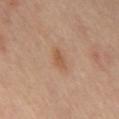Clinical impression:
This lesion was catalogued during total-body skin photography and was not selected for biopsy.
Context:
Captured under cross-polarized illumination. From the mid back. The lesion-visualizer software estimated a lesion area of about 4 mm², an outline eccentricity of about 0.85 (0 = round, 1 = elongated), and two-axis asymmetry of about 0.25. The software also gave a normalized border contrast of about 6. It also reported a border-irregularity rating of about 2.5/10, a color-variation rating of about 2.5/10, and a peripheral color-asymmetry measure near 1. The analysis additionally found a nevus-likeness score of about 50/100. The recorded lesion diameter is about 2.5 mm. A 15 mm close-up extracted from a 3D total-body photography capture.